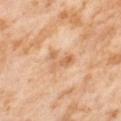The lesion was photographed on a routine skin check and not biopsied; there is no pathology result. This is a cross-polarized tile. A female patient, roughly 55 years of age. The recorded lesion diameter is about 3.5 mm. A 15 mm close-up tile from a total-body photography series done for melanoma screening. The lesion is on the right thigh. Automated tile analysis of the lesion measured a footprint of about 5 mm², an eccentricity of roughly 0.75, and two-axis asymmetry of about 0.5. The software also gave a mean CIELAB color near L≈66 a*≈22 b*≈38 and a lesion–skin lightness drop of about 9. It also reported an automated nevus-likeness rating near 0 out of 100.